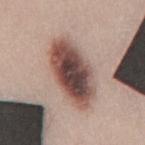Impression: Imaged during a routine full-body skin examination; the lesion was not biopsied and no histopathology is available. Context: The lesion's longest dimension is about 8 mm. Imaged with white-light lighting. A close-up tile cropped from a whole-body skin photograph, about 15 mm across. Located on the mid back. The total-body-photography lesion software estimated a lesion–skin lightness drop of about 20 and a lesion-to-skin contrast of about 13 (normalized; higher = more distinct). And it measured a border-irregularity index near 2/10, internal color variation of about 9.5 on a 0–10 scale, and a peripheral color-asymmetry measure near 3. A male patient about 45 years old.